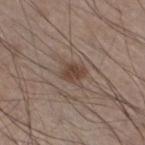Cropped from a total-body skin-imaging series; the visible field is about 15 mm.
The subject is a male aged 58 to 62.
From the left lower leg.
An algorithmic analysis of the crop reported a footprint of about 6 mm², an outline eccentricity of about 0.55 (0 = round, 1 = elongated), and a shape-asymmetry score of about 0.25 (0 = symmetric). And it measured an average lesion color of about L≈44 a*≈15 b*≈24 (CIELAB), about 9 CIELAB-L* units darker than the surrounding skin, and a normalized lesion–skin contrast near 7.5. The software also gave a border-irregularity index near 2.5/10, internal color variation of about 2.5 on a 0–10 scale, and a peripheral color-asymmetry measure near 1.